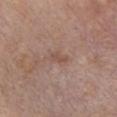Q: Was a biopsy performed?
A: imaged on a skin check; not biopsied
Q: What did automated image analysis measure?
A: a normalized border contrast of about 5.5
Q: What is the anatomic site?
A: the chest
Q: How was the tile lit?
A: white-light illumination
Q: What is the lesion's diameter?
A: ~2.5 mm (longest diameter)
Q: What is the imaging modality?
A: ~15 mm crop, total-body skin-cancer survey
Q: Who is the patient?
A: male, in their 60s